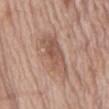{
  "biopsy_status": "not biopsied; imaged during a skin examination",
  "automated_metrics": {
    "eccentricity": 0.95,
    "shape_asymmetry": 0.3
  },
  "image": {
    "source": "total-body photography crop",
    "field_of_view_mm": 15
  },
  "lesion_size": {
    "long_diameter_mm_approx": 6.5
  },
  "patient": {
    "sex": "male",
    "age_approx": 70
  },
  "site": "abdomen",
  "lighting": "white-light"
}